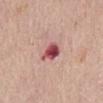<record>
  <biopsy_status>not biopsied; imaged during a skin examination</biopsy_status>
  <image>
    <source>total-body photography crop</source>
    <field_of_view_mm>15</field_of_view_mm>
  </image>
  <lesion_size>
    <long_diameter_mm_approx>3.0</long_diameter_mm_approx>
  </lesion_size>
  <patient>
    <sex>female</sex>
    <age_approx>65</age_approx>
  </patient>
  <site>abdomen</site>
  <automated_metrics>
    <cielab_L>50</cielab_L>
    <cielab_a>32</cielab_a>
    <cielab_b>21</cielab_b>
    <vs_skin_contrast_norm>12.0</vs_skin_contrast_norm>
    <border_irregularity_0_10>2.5</border_irregularity_0_10>
    <color_variation_0_10>6.0</color_variation_0_10>
    <peripheral_color_asymmetry>2.0</peripheral_color_asymmetry>
    <lesion_detection_confidence_0_100>100</lesion_detection_confidence_0_100>
  </automated_metrics>
</record>